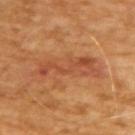Imaged during a routine full-body skin examination; the lesion was not biopsied and no histopathology is available. This is a cross-polarized tile. The total-body-photography lesion software estimated an area of roughly 17 mm² and a symmetry-axis asymmetry near 0.3. The software also gave a lesion color around L≈49 a*≈26 b*≈36 in CIELAB, roughly 7 lightness units darker than nearby skin, and a lesion-to-skin contrast of about 5.5 (normalized; higher = more distinct). And it measured a classifier nevus-likeness of about 0/100 and lesion-presence confidence of about 100/100. A 15 mm close-up extracted from a 3D total-body photography capture. Approximately 7.5 mm at its widest. A male patient, aged around 65.